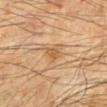No biopsy was performed on this lesion — it was imaged during a full skin examination and was not determined to be concerning. A male patient, about 70 years old. Located on the left forearm. A close-up tile cropped from a whole-body skin photograph, about 15 mm across.